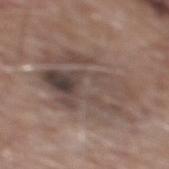No biopsy was performed on this lesion — it was imaged during a full skin examination and was not determined to be concerning.
The recorded lesion diameter is about 8.5 mm.
On the mid back.
Captured under white-light illumination.
A 15 mm close-up tile from a total-body photography series done for melanoma screening.
A male subject, about 60 years old.